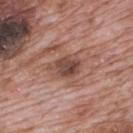Automated image analysis of the tile measured a lesion color around L≈47 a*≈21 b*≈25 in CIELAB, a lesion–skin lightness drop of about 12, and a normalized lesion–skin contrast near 8.5. The analysis additionally found a border-irregularity rating of about 2/10, internal color variation of about 6 on a 0–10 scale, and peripheral color asymmetry of about 2.
From the mid back.
This image is a 15 mm lesion crop taken from a total-body photograph.
A male subject, roughly 70 years of age.
The recorded lesion diameter is about 4 mm.